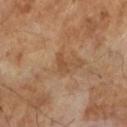Assessment:
The lesion was tiled from a total-body skin photograph and was not biopsied.
Clinical summary:
Imaged with cross-polarized lighting. A male subject, aged 63 to 67. This image is a 15 mm lesion crop taken from a total-body photograph. The recorded lesion diameter is about 2.5 mm.